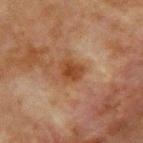Q: Is there a histopathology result?
A: imaged on a skin check; not biopsied
Q: What kind of image is this?
A: ~15 mm crop, total-body skin-cancer survey
Q: Patient demographics?
A: male, in their mid- to late 60s
Q: Where on the body is the lesion?
A: the chest
Q: Automated lesion metrics?
A: a footprint of about 5.5 mm² and a shape-asymmetry score of about 0.3 (0 = symmetric); a lesion-detection confidence of about 100/100
Q: How large is the lesion?
A: ~2.5 mm (longest diameter)
Q: Illumination type?
A: cross-polarized illumination